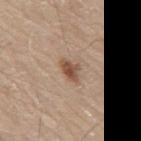biopsy status: total-body-photography surveillance lesion; no biopsy | lesion diameter: ≈3 mm | subject: male, aged around 80 | location: the mid back | image-analysis metrics: a border-irregularity rating of about 2.5/10, a within-lesion color-variation index near 3.5/10, and radial color variation of about 1 | image source: total-body-photography crop, ~15 mm field of view.